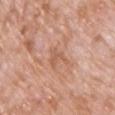Findings:
- biopsy status: catalogued during a skin exam; not biopsied
- size: ~3.5 mm (longest diameter)
- lighting: white-light illumination
- image: 15 mm crop, total-body photography
- patient: male, about 70 years old
- anatomic site: the chest
- TBP lesion metrics: an area of roughly 4 mm² and a shape eccentricity near 0.75; a mean CIELAB color near L≈58 a*≈23 b*≈32, about 8 CIELAB-L* units darker than the surrounding skin, and a lesion-to-skin contrast of about 5.5 (normalized; higher = more distinct)